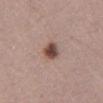Assessment: This lesion was catalogued during total-body skin photography and was not selected for biopsy. Clinical summary: The total-body-photography lesion software estimated a border-irregularity index near 2/10, a within-lesion color-variation index near 5.5/10, and radial color variation of about 1.5. The analysis additionally found a nevus-likeness score of about 95/100 and a lesion-detection confidence of about 100/100. Measured at roughly 3.5 mm in maximum diameter. This image is a 15 mm lesion crop taken from a total-body photograph. A female patient, aged around 40. Located on the mid back. The tile uses white-light illumination.